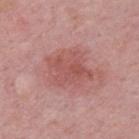biopsy status = catalogued during a skin exam; not biopsied | site = the upper back | tile lighting = white-light | automated metrics = border irregularity of about 3.5 on a 0–10 scale and a color-variation rating of about 3.5/10 | image source = ~15 mm crop, total-body skin-cancer survey | subject = male, roughly 50 years of age | size = ≈4.5 mm.